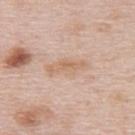workup = catalogued during a skin exam; not biopsied
size = about 5 mm
image source = ~15 mm crop, total-body skin-cancer survey
patient = female, aged around 65
site = the upper back